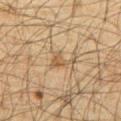Clinical impression: Captured during whole-body skin photography for melanoma surveillance; the lesion was not biopsied. Background: Measured at roughly 3 mm in maximum diameter. Automated image analysis of the tile measured a lesion color around L≈46 a*≈13 b*≈30 in CIELAB, a lesion–skin lightness drop of about 8, and a normalized border contrast of about 6.5. And it measured a border-irregularity rating of about 7/10, a color-variation rating of about 1/10, and peripheral color asymmetry of about 0.5. The tile uses cross-polarized illumination. Located on the front of the torso. A roughly 15 mm field-of-view crop from a total-body skin photograph. A male subject, in their mid- to late 60s.